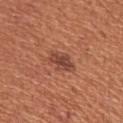biopsy_status: not biopsied; imaged during a skin examination
lighting: white-light
patient:
  sex: female
  age_approx: 65
site: left upper arm
image:
  source: total-body photography crop
  field_of_view_mm: 15